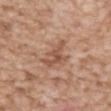No biopsy was performed on this lesion — it was imaged during a full skin examination and was not determined to be concerning. Longest diameter approximately 4 mm. Cropped from a whole-body photographic skin survey; the tile spans about 15 mm. A male subject roughly 60 years of age. On the chest.